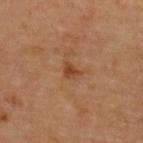{"biopsy_status": "not biopsied; imaged during a skin examination", "lesion_size": {"long_diameter_mm_approx": 2.5}, "lighting": "cross-polarized", "patient": {"sex": "female", "age_approx": 70}, "site": "upper back", "image": {"source": "total-body photography crop", "field_of_view_mm": 15}}